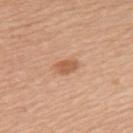biopsy status — total-body-photography surveillance lesion; no biopsy
body site — the left upper arm
subject — female, aged 38–42
image-analysis metrics — a footprint of about 4.5 mm², an eccentricity of roughly 0.8, and two-axis asymmetry of about 0.25; a normalized border contrast of about 7
lesion diameter — ≈3 mm
illumination — white-light
imaging modality — ~15 mm crop, total-body skin-cancer survey